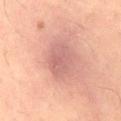Q: Lesion location?
A: the lower back
Q: Who is the patient?
A: male, aged 38 to 42
Q: How was the tile lit?
A: cross-polarized
Q: How was this image acquired?
A: ~15 mm crop, total-body skin-cancer survey